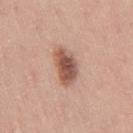Clinical impression: Imaged during a routine full-body skin examination; the lesion was not biopsied and no histopathology is available. Acquisition and patient details: From the right thigh. Cropped from a total-body skin-imaging series; the visible field is about 15 mm. About 4.5 mm across. A female subject, aged approximately 45.